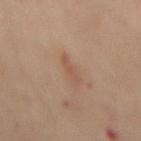workup = catalogued during a skin exam; not biopsied | location = the mid back | subject = female, about 60 years old | imaging modality = total-body-photography crop, ~15 mm field of view.